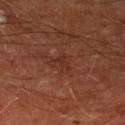Notes:
* follow-up: total-body-photography surveillance lesion; no biopsy
* anatomic site: the left lower leg
* lesion size: ~2.5 mm (longest diameter)
* illumination: cross-polarized
* image: total-body-photography crop, ~15 mm field of view
* subject: male, in their mid-60s